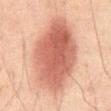The lesion was photographed on a routine skin check and not biopsied; there is no pathology result. Automated image analysis of the tile measured a border-irregularity index near 1.5/10 and a color-variation rating of about 4.5/10. And it measured a lesion-detection confidence of about 100/100. A male subject, in their mid- to late 60s. From the mid back. Measured at roughly 10 mm in maximum diameter. A lesion tile, about 15 mm wide, cut from a 3D total-body photograph. Imaged with cross-polarized lighting.